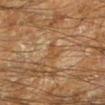Captured during whole-body skin photography for melanoma surveillance; the lesion was not biopsied.
A roughly 15 mm field-of-view crop from a total-body skin photograph.
The tile uses cross-polarized illumination.
The lesion is on the left lower leg.
Longest diameter approximately 2.5 mm.
A male subject in their 60s.